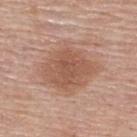Notes:
- location: the upper back
- tile lighting: white-light
- diameter: ~6.5 mm (longest diameter)
- image source: total-body-photography crop, ~15 mm field of view
- subject: female, aged 63 to 67